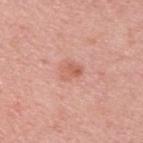Q: Was a biopsy performed?
A: total-body-photography surveillance lesion; no biopsy
Q: Automated lesion metrics?
A: an area of roughly 4.5 mm², an outline eccentricity of about 0.6 (0 = round, 1 = elongated), and two-axis asymmetry of about 0.35; an average lesion color of about L≈61 a*≈26 b*≈30 (CIELAB), about 8 CIELAB-L* units darker than the surrounding skin, and a normalized border contrast of about 6; border irregularity of about 3 on a 0–10 scale, internal color variation of about 4.5 on a 0–10 scale, and a peripheral color-asymmetry measure near 1.5; a lesion-detection confidence of about 100/100
Q: Illumination type?
A: white-light illumination
Q: Lesion location?
A: the back
Q: What is the imaging modality?
A: ~15 mm crop, total-body skin-cancer survey
Q: Patient demographics?
A: male, aged approximately 65
Q: How large is the lesion?
A: ≈2.5 mm